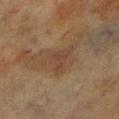This lesion was catalogued during total-body skin photography and was not selected for biopsy.
The lesion-visualizer software estimated a nevus-likeness score of about 5/100.
Located on the right lower leg.
The patient is a male aged 68–72.
Cropped from a total-body skin-imaging series; the visible field is about 15 mm.
The tile uses cross-polarized illumination.
Measured at roughly 3 mm in maximum diameter.